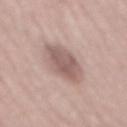notes: catalogued during a skin exam; not biopsied | imaging modality: total-body-photography crop, ~15 mm field of view | size: ~6 mm (longest diameter) | lighting: white-light | patient: male, aged approximately 60 | body site: the mid back.